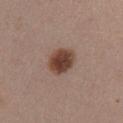Context:
Captured under white-light illumination. This image is a 15 mm lesion crop taken from a total-body photograph. An algorithmic analysis of the crop reported a border-irregularity index near 1.5/10, internal color variation of about 4 on a 0–10 scale, and peripheral color asymmetry of about 1. The lesion is located on the right upper arm. A female subject, approximately 30 years of age.